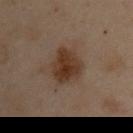Cropped from a whole-body photographic skin survey; the tile spans about 15 mm.
The lesion is located on the arm.
An algorithmic analysis of the crop reported a classifier nevus-likeness of about 85/100.
Measured at roughly 4.5 mm in maximum diameter.
A male patient aged 53–57.
The tile uses cross-polarized illumination.